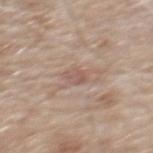Assessment:
Captured during whole-body skin photography for melanoma surveillance; the lesion was not biopsied.
Context:
The lesion is located on the mid back. A 15 mm close-up extracted from a 3D total-body photography capture. Imaged with white-light lighting. A male subject aged 78–82.